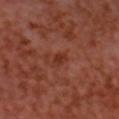Captured during whole-body skin photography for melanoma surveillance; the lesion was not biopsied. From the head or neck. A region of skin cropped from a whole-body photographic capture, roughly 15 mm wide. This is a cross-polarized tile. The patient is a male approximately 55 years of age.